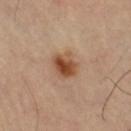Impression:
No biopsy was performed on this lesion — it was imaged during a full skin examination and was not determined to be concerning.
Background:
An algorithmic analysis of the crop reported a footprint of about 7.5 mm² and a shape eccentricity near 0.6. The analysis additionally found a lesion color around L≈50 a*≈22 b*≈33 in CIELAB and a normalized lesion–skin contrast near 9.5. A 15 mm crop from a total-body photograph taken for skin-cancer surveillance. On the right thigh. Approximately 3.5 mm at its widest.